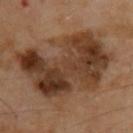Impression: Imaged during a routine full-body skin examination; the lesion was not biopsied and no histopathology is available. Context: Captured under cross-polarized illumination. The patient is a male in their 70s. A 15 mm close-up tile from a total-body photography series done for melanoma screening. The lesion is located on the upper back. The recorded lesion diameter is about 11.5 mm. Automated tile analysis of the lesion measured an area of roughly 50 mm² and a symmetry-axis asymmetry near 0.35. The software also gave a lesion color around L≈36 a*≈18 b*≈28 in CIELAB, about 12 CIELAB-L* units darker than the surrounding skin, and a normalized lesion–skin contrast near 10.5. The analysis additionally found a border-irregularity rating of about 5.5/10, internal color variation of about 7.5 on a 0–10 scale, and peripheral color asymmetry of about 2.5. It also reported a classifier nevus-likeness of about 0/100 and a detector confidence of about 100 out of 100 that the crop contains a lesion.